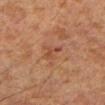Q: Was a biopsy performed?
A: no biopsy performed (imaged during a skin exam)
Q: What are the patient's age and sex?
A: male, roughly 65 years of age
Q: How was this image acquired?
A: total-body-photography crop, ~15 mm field of view
Q: Automated lesion metrics?
A: a lesion area of about 3 mm², a shape eccentricity near 0.9, and a shape-asymmetry score of about 0.45 (0 = symmetric); an average lesion color of about L≈42 a*≈23 b*≈30 (CIELAB), about 7 CIELAB-L* units darker than the surrounding skin, and a normalized border contrast of about 5.5; a nevus-likeness score of about 0/100 and a lesion-detection confidence of about 100/100
Q: How large is the lesion?
A: ≈3 mm
Q: Lesion location?
A: the arm